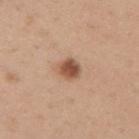Q: Was a biopsy performed?
A: no biopsy performed (imaged during a skin exam)
Q: What are the patient's age and sex?
A: male, aged around 40
Q: What is the anatomic site?
A: the upper back
Q: How was this image acquired?
A: ~15 mm crop, total-body skin-cancer survey